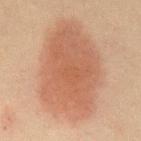| field | value |
|---|---|
| workup | no biopsy performed (imaged during a skin exam) |
| diameter | ≈10.5 mm |
| image source | total-body-photography crop, ~15 mm field of view |
| anatomic site | the abdomen |
| lighting | cross-polarized illumination |
| patient | male, about 60 years old |